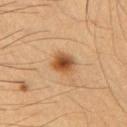Case summary:
- workup: catalogued during a skin exam; not biopsied
- patient: male, approximately 55 years of age
- image-analysis metrics: a mean CIELAB color near L≈45 a*≈19 b*≈34, about 12 CIELAB-L* units darker than the surrounding skin, and a lesion-to-skin contrast of about 9.5 (normalized; higher = more distinct); a border-irregularity rating of about 2/10, a color-variation rating of about 6.5/10, and radial color variation of about 2; an automated nevus-likeness rating near 100 out of 100 and lesion-presence confidence of about 100/100
- anatomic site: the left upper arm
- illumination: cross-polarized
- image source: 15 mm crop, total-body photography
- lesion diameter: about 3 mm